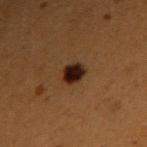Assessment:
Imaged during a routine full-body skin examination; the lesion was not biopsied and no histopathology is available.
Context:
The lesion is located on the right upper arm. The lesion's longest dimension is about 3 mm. A male patient aged around 50. A close-up tile cropped from a whole-body skin photograph, about 15 mm across. This is a cross-polarized tile. Automated tile analysis of the lesion measured a footprint of about 5.5 mm², an outline eccentricity of about 0.5 (0 = round, 1 = elongated), and a shape-asymmetry score of about 0.15 (0 = symmetric). And it measured roughly 12 lightness units darker than nearby skin and a normalized lesion–skin contrast near 15.5. And it measured a classifier nevus-likeness of about 100/100 and a detector confidence of about 100 out of 100 that the crop contains a lesion.